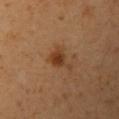This lesion was catalogued during total-body skin photography and was not selected for biopsy.
A lesion tile, about 15 mm wide, cut from a 3D total-body photograph.
The total-body-photography lesion software estimated a mean CIELAB color near L≈39 a*≈21 b*≈34, roughly 9 lightness units darker than nearby skin, and a normalized lesion–skin contrast near 8.
The patient is a female aged approximately 40.
On the arm.
Captured under cross-polarized illumination.
The recorded lesion diameter is about 3.5 mm.